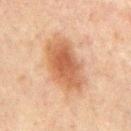{
  "biopsy_status": "not biopsied; imaged during a skin examination",
  "lesion_size": {
    "long_diameter_mm_approx": 6.0
  },
  "site": "mid back",
  "image": {
    "source": "total-body photography crop",
    "field_of_view_mm": 15
  },
  "patient": {
    "sex": "male",
    "age_approx": 65
  }
}